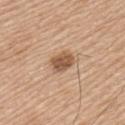follow-up = catalogued during a skin exam; not biopsied
patient = male, in their mid-70s
automated lesion analysis = a shape eccentricity near 0.6 and a symmetry-axis asymmetry near 0.2; a normalized border contrast of about 9; a color-variation rating of about 3.5/10 and radial color variation of about 1.5
body site = the arm
acquisition = ~15 mm crop, total-body skin-cancer survey
diameter = ~3 mm (longest diameter)